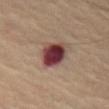| feature | finding |
|---|---|
| body site | the chest |
| tile lighting | cross-polarized illumination |
| image | ~15 mm crop, total-body skin-cancer survey |
| subject | male, roughly 70 years of age |
| lesion diameter | ≈4 mm |